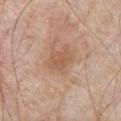biopsy status: no biopsy performed (imaged during a skin exam)
automated lesion analysis: a mean CIELAB color near L≈56 a*≈20 b*≈32, roughly 8 lightness units darker than nearby skin, and a lesion-to-skin contrast of about 5.5 (normalized; higher = more distinct)
diameter: about 3 mm
tile lighting: white-light
location: the chest
patient: male, aged approximately 80
image source: total-body-photography crop, ~15 mm field of view